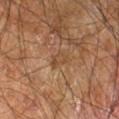follow-up: no biopsy performed (imaged during a skin exam)
diameter: about 2.5 mm
site: the right leg
automated lesion analysis: a lesion area of about 3.5 mm² and an eccentricity of roughly 0.75; a border-irregularity rating of about 2.5/10, a color-variation rating of about 2/10, and radial color variation of about 0.5; a nevus-likeness score of about 0/100 and a detector confidence of about 95 out of 100 that the crop contains a lesion
patient: male, roughly 60 years of age
tile lighting: cross-polarized
image source: total-body-photography crop, ~15 mm field of view